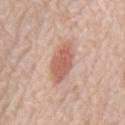Captured during whole-body skin photography for melanoma surveillance; the lesion was not biopsied. The subject is a male aged 78–82. A region of skin cropped from a whole-body photographic capture, roughly 15 mm wide. On the back. The lesion-visualizer software estimated an area of roughly 10 mm², a shape eccentricity near 0.75, and two-axis asymmetry of about 0.15. And it measured a lesion color around L≈60 a*≈23 b*≈28 in CIELAB, about 11 CIELAB-L* units darker than the surrounding skin, and a lesion-to-skin contrast of about 7.5 (normalized; higher = more distinct). It also reported an automated nevus-likeness rating near 100 out of 100 and a detector confidence of about 100 out of 100 that the crop contains a lesion.